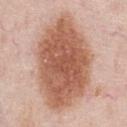No biopsy was performed on this lesion — it was imaged during a full skin examination and was not determined to be concerning.
On the chest.
The tile uses white-light illumination.
A region of skin cropped from a whole-body photographic capture, roughly 15 mm wide.
Automated tile analysis of the lesion measured a footprint of about 55 mm², a shape eccentricity near 0.8, and a shape-asymmetry score of about 0.15 (0 = symmetric). And it measured border irregularity of about 2 on a 0–10 scale, a color-variation rating of about 4.5/10, and radial color variation of about 1.5. And it measured a classifier nevus-likeness of about 100/100 and lesion-presence confidence of about 100/100.
The lesion's longest dimension is about 11.5 mm.
A male subject about 60 years old.